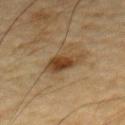biopsy_status: not biopsied; imaged during a skin examination
lighting: cross-polarized
site: front of the torso
image:
  source: total-body photography crop
  field_of_view_mm: 15
automated_metrics:
  border_irregularity_0_10: 2.5
  peripheral_color_asymmetry: 1.5
lesion_size:
  long_diameter_mm_approx: 4.0
patient:
  sex: male
  age_approx: 85+